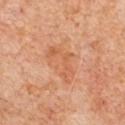The lesion was tiled from a total-body skin photograph and was not biopsied. A male subject in their 60s. Located on the front of the torso. Cropped from a whole-body photographic skin survey; the tile spans about 15 mm. The lesion's longest dimension is about 5 mm.